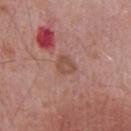Impression: Captured during whole-body skin photography for melanoma surveillance; the lesion was not biopsied. Image and clinical context: The patient is a male aged 68–72. About 2.5 mm across. Imaged with white-light lighting. A roughly 15 mm field-of-view crop from a total-body skin photograph. The total-body-photography lesion software estimated an area of roughly 4 mm², a shape eccentricity near 0.6, and two-axis asymmetry of about 0.25. The software also gave internal color variation of about 2 on a 0–10 scale. The analysis additionally found a nevus-likeness score of about 0/100. On the chest.